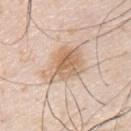Part of a total-body skin-imaging series; this lesion was reviewed on a skin check and was not flagged for biopsy.
A roughly 15 mm field-of-view crop from a total-body skin photograph.
Approximately 6 mm at its widest.
On the upper back.
A male patient aged approximately 40.
This is a white-light tile.